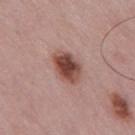  biopsy_status: not biopsied; imaged during a skin examination
  site: mid back
  patient:
    sex: male
    age_approx: 50
  image:
    source: total-body photography crop
    field_of_view_mm: 15
  lesion_size:
    long_diameter_mm_approx: 4.0
  automated_metrics:
    area_mm2_approx: 9.0
    eccentricity: 0.7
    shape_asymmetry: 0.15
    cielab_L: 46
    cielab_a: 22
    cielab_b: 24
    vs_skin_darker_L: 16.0
    vs_skin_contrast_norm: 11.5
    border_irregularity_0_10: 1.5
    color_variation_0_10: 6.5
    peripheral_color_asymmetry: 1.5
    nevus_likeness_0_100: 95
    lesion_detection_confidence_0_100: 100